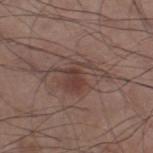Captured under white-light illumination.
From the left thigh.
A male patient, in their 60s.
Automated tile analysis of the lesion measured a shape eccentricity near 0.8 and two-axis asymmetry of about 0.45. The analysis additionally found roughly 8 lightness units darker than nearby skin and a normalized border contrast of about 6.5. The software also gave lesion-presence confidence of about 100/100.
Approximately 6.5 mm at its widest.
A roughly 15 mm field-of-view crop from a total-body skin photograph.